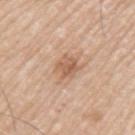{"biopsy_status": "not biopsied; imaged during a skin examination", "lighting": "white-light", "patient": {"sex": "male", "age_approx": 65}, "lesion_size": {"long_diameter_mm_approx": 3.0}, "image": {"source": "total-body photography crop", "field_of_view_mm": 15}, "site": "left upper arm"}